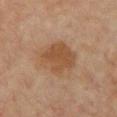The lesion was tiled from a total-body skin photograph and was not biopsied. The tile uses cross-polarized illumination. The subject is a female aged approximately 70. Automated image analysis of the tile measured a border-irregularity index near 2/10 and radial color variation of about 1. And it measured a nevus-likeness score of about 40/100. Located on the chest. A 15 mm crop from a total-body photograph taken for skin-cancer surveillance.